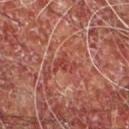Acquisition and patient details:
A close-up tile cropped from a whole-body skin photograph, about 15 mm across. The lesion is located on the chest. A male subject aged around 55. Automated tile analysis of the lesion measured a classifier nevus-likeness of about 0/100 and lesion-presence confidence of about 75/100. Longest diameter approximately 2.5 mm.